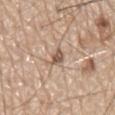<lesion>
  <biopsy_status>not biopsied; imaged during a skin examination</biopsy_status>
  <lesion_size>
    <long_diameter_mm_approx>2.5</long_diameter_mm_approx>
  </lesion_size>
  <automated_metrics>
    <area_mm2_approx>3.0</area_mm2_approx>
    <eccentricity>0.75</eccentricity>
    <shape_asymmetry>0.35</shape_asymmetry>
    <lesion_detection_confidence_0_100>95</lesion_detection_confidence_0_100>
  </automated_metrics>
  <lighting>white-light</lighting>
  <site>mid back</site>
  <patient>
    <sex>male</sex>
    <age_approx>80</age_approx>
  </patient>
  <image>
    <source>total-body photography crop</source>
    <field_of_view_mm>15</field_of_view_mm>
  </image>
</lesion>